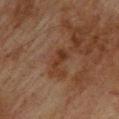notes: total-body-photography surveillance lesion; no biopsy
diameter: ≈2.5 mm
automated metrics: a classifier nevus-likeness of about 0/100
site: the upper back
acquisition: ~15 mm tile from a whole-body skin photo
lighting: cross-polarized
patient: male, in their mid- to late 70s